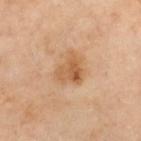Part of a total-body skin-imaging series; this lesion was reviewed on a skin check and was not flagged for biopsy. About 3.5 mm across. A 15 mm crop from a total-body photograph taken for skin-cancer surveillance. A female subject, in their mid-50s. From the left thigh.